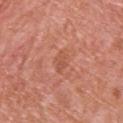Assessment:
The lesion was tiled from a total-body skin photograph and was not biopsied.
Image and clinical context:
The patient is a female aged approximately 40. Cropped from a total-body skin-imaging series; the visible field is about 15 mm. The tile uses white-light illumination. Measured at roughly 3 mm in maximum diameter. From the upper back.